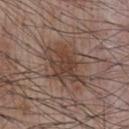| key | value |
|---|---|
| lesion size | ~6.5 mm (longest diameter) |
| subject | male, approximately 55 years of age |
| lighting | white-light illumination |
| site | the front of the torso |
| acquisition | ~15 mm crop, total-body skin-cancer survey |